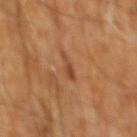Imaged during a routine full-body skin examination; the lesion was not biopsied and no histopathology is available.